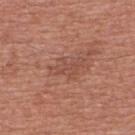Clinical impression:
Imaged during a routine full-body skin examination; the lesion was not biopsied and no histopathology is available.
Acquisition and patient details:
Measured at roughly 4 mm in maximum diameter. The lesion is located on the chest. A 15 mm crop from a total-body photograph taken for skin-cancer surveillance. The lesion-visualizer software estimated a lesion color around L≈49 a*≈24 b*≈28 in CIELAB, a lesion–skin lightness drop of about 6, and a lesion-to-skin contrast of about 5 (normalized; higher = more distinct). And it measured an automated nevus-likeness rating near 0 out of 100 and a detector confidence of about 95 out of 100 that the crop contains a lesion. The patient is a male in their mid- to late 40s. The tile uses white-light illumination.